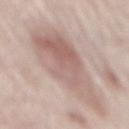Clinical impression:
Recorded during total-body skin imaging; not selected for excision or biopsy.
Clinical summary:
The lesion is on the back. This is a white-light tile. A close-up tile cropped from a whole-body skin photograph, about 15 mm across. An algorithmic analysis of the crop reported an area of roughly 38 mm², a shape eccentricity near 0.9, and a symmetry-axis asymmetry near 0.3. And it measured a border-irregularity rating of about 4/10, a color-variation rating of about 5/10, and peripheral color asymmetry of about 1.5. The analysis additionally found lesion-presence confidence of about 55/100. The subject is a male roughly 80 years of age.